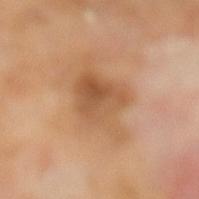Notes:
• workup · total-body-photography surveillance lesion; no biopsy
• subject · male, roughly 65 years of age
• imaging modality · ~15 mm crop, total-body skin-cancer survey
• automated metrics · a lesion–skin lightness drop of about 10 and a lesion-to-skin contrast of about 7 (normalized; higher = more distinct)
• illumination · cross-polarized
• body site · the left lower leg